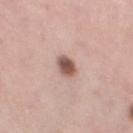Assessment:
Imaged during a routine full-body skin examination; the lesion was not biopsied and no histopathology is available.
Clinical summary:
The lesion-visualizer software estimated an area of roughly 5 mm², an outline eccentricity of about 0.35 (0 = round, 1 = elongated), and two-axis asymmetry of about 0.15. The analysis additionally found a mean CIELAB color near L≈55 a*≈20 b*≈24, about 16 CIELAB-L* units darker than the surrounding skin, and a lesion-to-skin contrast of about 10 (normalized; higher = more distinct). The software also gave a border-irregularity rating of about 1.5/10, internal color variation of about 5 on a 0–10 scale, and radial color variation of about 1.5. The software also gave a nevus-likeness score of about 95/100. A lesion tile, about 15 mm wide, cut from a 3D total-body photograph. Imaged with white-light lighting. A female patient, about 50 years old. Located on the left thigh. The lesion's longest dimension is about 2.5 mm.